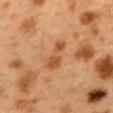Q: Lesion location?
A: the mid back
Q: What are the patient's age and sex?
A: female, approximately 40 years of age
Q: What is the lesion's diameter?
A: about 4 mm
Q: What lighting was used for the tile?
A: cross-polarized illumination
Q: How was this image acquired?
A: ~15 mm crop, total-body skin-cancer survey